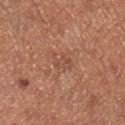Q: What kind of image is this?
A: 15 mm crop, total-body photography
Q: What did automated image analysis measure?
A: a lesion area of about 3.5 mm², a shape eccentricity near 0.8, and two-axis asymmetry of about 0.35; a lesion color around L≈49 a*≈23 b*≈30 in CIELAB, about 6 CIELAB-L* units darker than the surrounding skin, and a normalized border contrast of about 4.5; a lesion-detection confidence of about 100/100
Q: Lesion location?
A: the right lower leg
Q: Patient demographics?
A: male, roughly 70 years of age
Q: How was the tile lit?
A: white-light
Q: How large is the lesion?
A: ~2.5 mm (longest diameter)